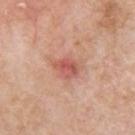notes = total-body-photography surveillance lesion; no biopsy.